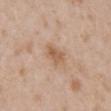<tbp_lesion>
  <biopsy_status>not biopsied; imaged during a skin examination</biopsy_status>
  <image>
    <source>total-body photography crop</source>
    <field_of_view_mm>15</field_of_view_mm>
  </image>
  <site>mid back</site>
  <lighting>white-light</lighting>
  <lesion_size>
    <long_diameter_mm_approx>3.0</long_diameter_mm_approx>
  </lesion_size>
  <patient>
    <sex>female</sex>
    <age_approx>30</age_approx>
  </patient>
</tbp_lesion>